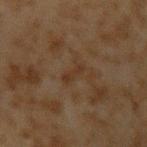Q: Is there a histopathology result?
A: catalogued during a skin exam; not biopsied
Q: What kind of image is this?
A: total-body-photography crop, ~15 mm field of view
Q: How was the tile lit?
A: cross-polarized illumination
Q: Lesion location?
A: the left upper arm
Q: What did automated image analysis measure?
A: a shape-asymmetry score of about 0.35 (0 = symmetric); a mean CIELAB color near L≈26 a*≈13 b*≈24 and a normalized lesion–skin contrast near 5.5; a within-lesion color-variation index near 0/10 and peripheral color asymmetry of about 0; a classifier nevus-likeness of about 0/100 and a detector confidence of about 100 out of 100 that the crop contains a lesion
Q: Patient demographics?
A: male, approximately 45 years of age